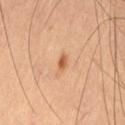Captured during whole-body skin photography for melanoma surveillance; the lesion was not biopsied.